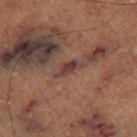The lesion was tiled from a total-body skin photograph and was not biopsied. An algorithmic analysis of the crop reported a lesion color around L≈29 a*≈17 b*≈17 in CIELAB, a lesion–skin lightness drop of about 8, and a normalized lesion–skin contrast near 9.5. And it measured border irregularity of about 3.5 on a 0–10 scale and internal color variation of about 1 on a 0–10 scale. This is a cross-polarized tile. The recorded lesion diameter is about 3 mm. A 15 mm close-up extracted from a 3D total-body photography capture. A male subject roughly 75 years of age. Located on the right thigh.